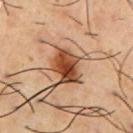workup: no biopsy performed (imaged during a skin exam)
subject: male, aged 53–57
location: the chest
image source: ~15 mm crop, total-body skin-cancer survey